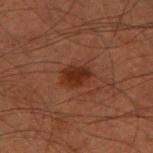Impression:
Imaged during a routine full-body skin examination; the lesion was not biopsied and no histopathology is available.
Acquisition and patient details:
The recorded lesion diameter is about 3 mm. This is a cross-polarized tile. The lesion is on the right upper arm. A male subject, aged 58 to 62. The lesion-visualizer software estimated an eccentricity of roughly 0.7. And it measured a lesion color around L≈22 a*≈20 b*≈24 in CIELAB and a normalized lesion–skin contrast near 9. It also reported border irregularity of about 1.5 on a 0–10 scale and peripheral color asymmetry of about 1. The analysis additionally found an automated nevus-likeness rating near 95 out of 100 and a detector confidence of about 100 out of 100 that the crop contains a lesion. A region of skin cropped from a whole-body photographic capture, roughly 15 mm wide.